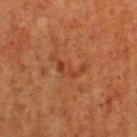Assessment:
This lesion was catalogued during total-body skin photography and was not selected for biopsy.
Context:
Automated tile analysis of the lesion measured a footprint of about 5 mm², an eccentricity of roughly 0.9, and a symmetry-axis asymmetry near 0.65. The analysis additionally found a mean CIELAB color near L≈35 a*≈23 b*≈32 and a normalized lesion–skin contrast near 5.5. The software also gave peripheral color asymmetry of about 0.5. The analysis additionally found a nevus-likeness score of about 0/100 and a lesion-detection confidence of about 100/100. A 15 mm crop from a total-body photograph taken for skin-cancer surveillance. On the right thigh. Captured under cross-polarized illumination. A female subject, aged 48 to 52.